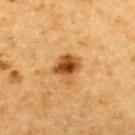A roughly 15 mm field-of-view crop from a total-body skin photograph. A male subject, aged around 85 or older. The tile uses cross-polarized illumination. Located on the upper back. An algorithmic analysis of the crop reported a lesion color around L≈45 a*≈21 b*≈40 in CIELAB, roughly 13 lightness units darker than nearby skin, and a lesion-to-skin contrast of about 10 (normalized; higher = more distinct). It also reported border irregularity of about 2.5 on a 0–10 scale, a color-variation rating of about 7/10, and radial color variation of about 2.5. It also reported a lesion-detection confidence of about 100/100.